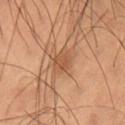{"biopsy_status": "not biopsied; imaged during a skin examination", "image": {"source": "total-body photography crop", "field_of_view_mm": 15}, "patient": {"sex": "male", "age_approx": 60}, "lighting": "cross-polarized", "site": "lower back", "lesion_size": {"long_diameter_mm_approx": 3.0}, "automated_metrics": {"cielab_L": 52, "cielab_a": 22, "cielab_b": 35, "border_irregularity_0_10": 1.5, "color_variation_0_10": 2.0, "peripheral_color_asymmetry": 0.5, "nevus_likeness_0_100": 40, "lesion_detection_confidence_0_100": 100}}